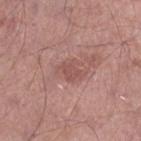Q: Is there a histopathology result?
A: no biopsy performed (imaged during a skin exam)
Q: Where on the body is the lesion?
A: the right lower leg
Q: How was the tile lit?
A: white-light
Q: What is the imaging modality?
A: 15 mm crop, total-body photography
Q: Who is the patient?
A: male, aged around 55